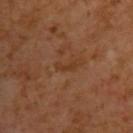Recorded during total-body skin imaging; not selected for excision or biopsy.
The lesion is located on the upper back.
A male subject, in their mid- to late 60s.
A roughly 15 mm field-of-view crop from a total-body skin photograph.
The lesion's longest dimension is about 2.5 mm.